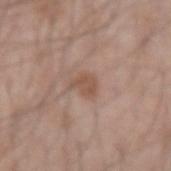Findings:
– workup · catalogued during a skin exam; not biopsied
– location · the left forearm
– patient · male, in their mid- to late 40s
– automated metrics · an area of roughly 4 mm²; a mean CIELAB color near L≈52 a*≈18 b*≈27, about 8 CIELAB-L* units darker than the surrounding skin, and a normalized lesion–skin contrast near 6.5
– acquisition · ~15 mm crop, total-body skin-cancer survey